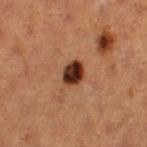{
  "biopsy_status": "not biopsied; imaged during a skin examination",
  "automated_metrics": {
    "area_mm2_approx": 5.5,
    "eccentricity": 0.65,
    "shape_asymmetry": 0.25,
    "cielab_L": 26,
    "cielab_a": 20,
    "cielab_b": 25,
    "vs_skin_darker_L": 18.0,
    "vs_skin_contrast_norm": 16.5,
    "nevus_likeness_0_100": 95,
    "lesion_detection_confidence_0_100": 100
  },
  "lighting": "cross-polarized",
  "patient": {
    "sex": "female",
    "age_approx": 40
  },
  "image": {
    "source": "total-body photography crop",
    "field_of_view_mm": 15
  },
  "site": "left thigh",
  "lesion_size": {
    "long_diameter_mm_approx": 2.5
  }
}